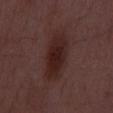Background: About 7 mm across. Captured under white-light illumination. A 15 mm crop from a total-body photograph taken for skin-cancer surveillance. The subject is a male in their 50s.